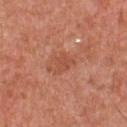The lesion is on the chest. Automated tile analysis of the lesion measured an average lesion color of about L≈51 a*≈27 b*≈34 (CIELAB), about 7 CIELAB-L* units darker than the surrounding skin, and a normalized border contrast of about 5.5. It also reported border irregularity of about 4 on a 0–10 scale and a color-variation rating of about 2/10. Imaged with white-light lighting. A male subject, about 55 years old. A 15 mm close-up extracted from a 3D total-body photography capture. About 3 mm across.